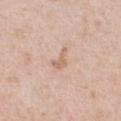Recorded during total-body skin imaging; not selected for excision or biopsy.